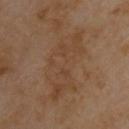subject: male, aged around 55 | site: the upper back | image: ~15 mm tile from a whole-body skin photo | automated metrics: a footprint of about 23 mm², an eccentricity of roughly 0.9, and a shape-asymmetry score of about 0.4 (0 = symmetric); a border-irregularity rating of about 6/10, a color-variation rating of about 3.5/10, and a peripheral color-asymmetry measure near 1; a classifier nevus-likeness of about 0/100 and lesion-presence confidence of about 100/100 | tile lighting: cross-polarized | lesion diameter: ≈9 mm.